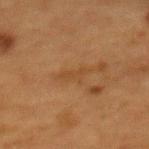Q: Was this lesion biopsied?
A: total-body-photography surveillance lesion; no biopsy
Q: What did automated image analysis measure?
A: an area of roughly 3.5 mm², an eccentricity of roughly 0.9, and a symmetry-axis asymmetry near 0.35; border irregularity of about 5 on a 0–10 scale, internal color variation of about 0 on a 0–10 scale, and peripheral color asymmetry of about 0; a nevus-likeness score of about 0/100
Q: What kind of image is this?
A: ~15 mm crop, total-body skin-cancer survey
Q: What lighting was used for the tile?
A: cross-polarized illumination
Q: Who is the patient?
A: female, aged 48–52
Q: How large is the lesion?
A: about 3.5 mm
Q: Lesion location?
A: the back